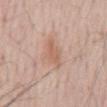body site: the mid back
subject: male, about 80 years old
automated lesion analysis: a lesion area of about 7 mm², a shape eccentricity near 0.8, and a symmetry-axis asymmetry near 0.25; a border-irregularity rating of about 3/10, a color-variation rating of about 1.5/10, and peripheral color asymmetry of about 0.5
image: 15 mm crop, total-body photography